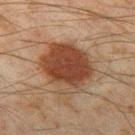biopsy status: total-body-photography surveillance lesion; no biopsy
location: the left thigh
patient: male, approximately 45 years of age
lesion size: about 6.5 mm
image source: ~15 mm crop, total-body skin-cancer survey